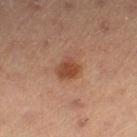Captured during whole-body skin photography for melanoma surveillance; the lesion was not biopsied.
Captured under cross-polarized illumination.
A 15 mm close-up extracted from a 3D total-body photography capture.
The subject is a male about 55 years old.
On the right lower leg.
Longest diameter approximately 3 mm.
The lesion-visualizer software estimated border irregularity of about 2 on a 0–10 scale and internal color variation of about 2.5 on a 0–10 scale.